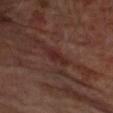Captured under cross-polarized illumination.
A male subject, in their mid-60s.
From the left upper arm.
The lesion's longest dimension is about 4 mm.
A lesion tile, about 15 mm wide, cut from a 3D total-body photograph.